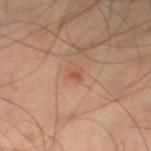No biopsy was performed on this lesion — it was imaged during a full skin examination and was not determined to be concerning. A male patient, in their mid-40s. The lesion-visualizer software estimated an eccentricity of roughly 0.7. The software also gave a classifier nevus-likeness of about 15/100. Cropped from a whole-body photographic skin survey; the tile spans about 15 mm. Imaged with cross-polarized lighting. The lesion is located on the leg.